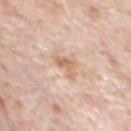Q: Was this lesion biopsied?
A: imaged on a skin check; not biopsied
Q: Automated lesion metrics?
A: a lesion color around L≈66 a*≈19 b*≈32 in CIELAB and a normalized border contrast of about 6.5
Q: What kind of image is this?
A: ~15 mm tile from a whole-body skin photo
Q: What is the anatomic site?
A: the chest
Q: What are the patient's age and sex?
A: male, roughly 80 years of age